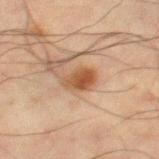Located on the left thigh.
A lesion tile, about 15 mm wide, cut from a 3D total-body photograph.
A male patient, aged around 65.